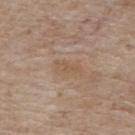Impression: Part of a total-body skin-imaging series; this lesion was reviewed on a skin check and was not flagged for biopsy. Acquisition and patient details: This image is a 15 mm lesion crop taken from a total-body photograph. Automated tile analysis of the lesion measured a footprint of about 3 mm² and a symmetry-axis asymmetry near 0.4. It also reported a lesion color around L≈54 a*≈16 b*≈30 in CIELAB, about 5 CIELAB-L* units darker than the surrounding skin, and a normalized lesion–skin contrast near 5. The lesion is on the upper back. A female subject, aged 73 to 77. This is a white-light tile. About 3 mm across.